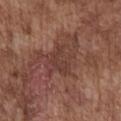Impression: Captured during whole-body skin photography for melanoma surveillance; the lesion was not biopsied. Image and clinical context: Approximately 4 mm at its widest. A region of skin cropped from a whole-body photographic capture, roughly 15 mm wide. This is a white-light tile. Located on the chest. The lesion-visualizer software estimated an eccentricity of roughly 0.75 and two-axis asymmetry of about 0.4. The software also gave a lesion–skin lightness drop of about 6. It also reported border irregularity of about 4 on a 0–10 scale, internal color variation of about 1.5 on a 0–10 scale, and peripheral color asymmetry of about 0.5. And it measured a nevus-likeness score of about 0/100. The patient is a male aged approximately 75.